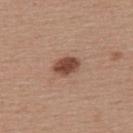Notes:
- notes — imaged on a skin check; not biopsied
- image source — ~15 mm tile from a whole-body skin photo
- location — the upper back
- patient — male, aged 53–57
- lesion size — ~3.5 mm (longest diameter)
- automated lesion analysis — an average lesion color of about L≈46 a*≈22 b*≈28 (CIELAB) and a lesion–skin lightness drop of about 14; a classifier nevus-likeness of about 100/100 and lesion-presence confidence of about 100/100